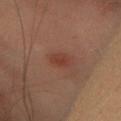| feature | finding |
|---|---|
| biopsy status | imaged on a skin check; not biopsied |
| TBP lesion metrics | a footprint of about 3.5 mm² and two-axis asymmetry of about 0.2; a mean CIELAB color near L≈30 a*≈19 b*≈23 and a lesion-to-skin contrast of about 6 (normalized; higher = more distinct); a border-irregularity index near 2/10, a within-lesion color-variation index near 1.5/10, and a peripheral color-asymmetry measure near 0.5; a classifier nevus-likeness of about 85/100 and lesion-presence confidence of about 100/100 |
| image source | ~15 mm tile from a whole-body skin photo |
| subject | female, aged 28 to 32 |
| body site | the head or neck |
| illumination | cross-polarized |
| lesion size | about 2.5 mm |